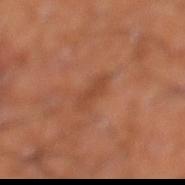{"site": "left lower leg", "lighting": "cross-polarized", "patient": {"sex": "male", "age_approx": 60}, "image": {"source": "total-body photography crop", "field_of_view_mm": 15}, "lesion_size": {"long_diameter_mm_approx": 3.0}, "automated_metrics": {"area_mm2_approx": 4.0, "shape_asymmetry": 0.25, "cielab_L": 44, "cielab_a": 24, "cielab_b": 32, "vs_skin_darker_L": 6.0, "vs_skin_contrast_norm": 5.0, "border_irregularity_0_10": 3.0, "color_variation_0_10": 1.0, "nevus_likeness_0_100": 0}}